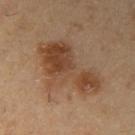No biopsy was performed on this lesion — it was imaged during a full skin examination and was not determined to be concerning. A male patient, aged 53 to 57. On the right upper arm. This image is a 15 mm lesion crop taken from a total-body photograph.